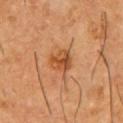Q: Was a biopsy performed?
A: catalogued during a skin exam; not biopsied
Q: Where on the body is the lesion?
A: the chest
Q: Who is the patient?
A: male, aged approximately 60
Q: What is the imaging modality?
A: ~15 mm crop, total-body skin-cancer survey